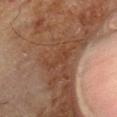workup = catalogued during a skin exam; not biopsied
automated metrics = a lesion area of about 5 mm², a shape eccentricity near 0.9, and a symmetry-axis asymmetry near 0.5; about 5 CIELAB-L* units darker than the surrounding skin and a normalized lesion–skin contrast near 5; an automated nevus-likeness rating near 0 out of 100 and a detector confidence of about 95 out of 100 that the crop contains a lesion
lesion diameter = about 4 mm
site = the left thigh
patient = male, approximately 80 years of age
image source = total-body-photography crop, ~15 mm field of view
tile lighting = cross-polarized